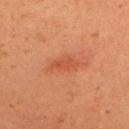| field | value |
|---|---|
| biopsy status | no biopsy performed (imaged during a skin exam) |
| patient | female, aged approximately 40 |
| lesion size | about 3 mm |
| automated metrics | a border-irregularity index near 3/10, a color-variation rating of about 0/10, and a peripheral color-asymmetry measure near 0 |
| image | total-body-photography crop, ~15 mm field of view |
| location | the upper back |